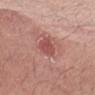Q: Was this lesion biopsied?
A: no biopsy performed (imaged during a skin exam)
Q: What lighting was used for the tile?
A: white-light
Q: Lesion size?
A: ~3 mm (longest diameter)
Q: Lesion location?
A: the abdomen
Q: What kind of image is this?
A: ~15 mm crop, total-body skin-cancer survey
Q: Who is the patient?
A: female, roughly 60 years of age